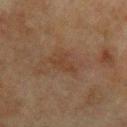– lesion size · ~3.5 mm (longest diameter)
– subject · male, in their mid- to late 70s
– anatomic site · the left upper arm
– image source · ~15 mm tile from a whole-body skin photo
– illumination · cross-polarized illumination
– automated lesion analysis · a lesion area of about 5.5 mm² and a symmetry-axis asymmetry near 0.4; a lesion–skin lightness drop of about 4 and a normalized border contrast of about 5